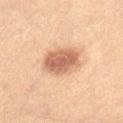Assessment:
Recorded during total-body skin imaging; not selected for excision or biopsy.
Context:
A male subject aged around 55. An algorithmic analysis of the crop reported an area of roughly 13 mm², an eccentricity of roughly 0.5, and a symmetry-axis asymmetry near 0.1. The analysis additionally found an average lesion color of about L≈60 a*≈22 b*≈32 (CIELAB), about 14 CIELAB-L* units darker than the surrounding skin, and a lesion-to-skin contrast of about 8.5 (normalized; higher = more distinct). Captured under cross-polarized illumination. A region of skin cropped from a whole-body photographic capture, roughly 15 mm wide. About 4 mm across. From the left thigh.